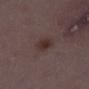Clinical impression:
This lesion was catalogued during total-body skin photography and was not selected for biopsy.